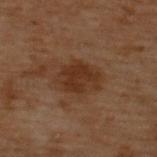The lesion was tiled from a total-body skin photograph and was not biopsied. This is a cross-polarized tile. A 15 mm close-up extracted from a 3D total-body photography capture. The lesion is on the upper back. Measured at roughly 4.5 mm in maximum diameter. The lesion-visualizer software estimated an area of roughly 12 mm² and a shape-asymmetry score of about 0.15 (0 = symmetric). The software also gave a color-variation rating of about 2.5/10 and peripheral color asymmetry of about 1. It also reported an automated nevus-likeness rating near 70 out of 100 and a detector confidence of about 100 out of 100 that the crop contains a lesion. A male subject approximately 70 years of age.